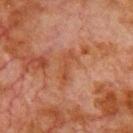Assessment:
Imaged during a routine full-body skin examination; the lesion was not biopsied and no histopathology is available.
Clinical summary:
The lesion-visualizer software estimated a lesion color around L≈38 a*≈22 b*≈29 in CIELAB, a lesion–skin lightness drop of about 5, and a normalized border contrast of about 5.5. The analysis additionally found an automated nevus-likeness rating near 0 out of 100 and a lesion-detection confidence of about 100/100. A roughly 15 mm field-of-view crop from a total-body skin photograph. The lesion is on the chest. Longest diameter approximately 4 mm. A male subject aged 78 to 82.